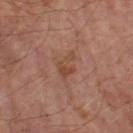Clinical impression: The lesion was photographed on a routine skin check and not biopsied; there is no pathology result. Context: A 15 mm crop from a total-body photograph taken for skin-cancer surveillance. On the left thigh. A male patient, aged 63–67. This is a cross-polarized tile. An algorithmic analysis of the crop reported a border-irregularity rating of about 5.5/10, a within-lesion color-variation index near 1.5/10, and radial color variation of about 0.5. And it measured an automated nevus-likeness rating near 0 out of 100 and lesion-presence confidence of about 100/100. The recorded lesion diameter is about 3.5 mm.